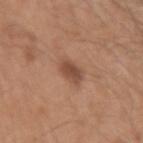The lesion was photographed on a routine skin check and not biopsied; there is no pathology result. On the left upper arm. Imaged with white-light lighting. The subject is a male aged around 50. A roughly 15 mm field-of-view crop from a total-body skin photograph.